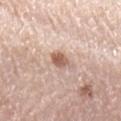Notes:
• biopsy status · no biopsy performed (imaged during a skin exam)
• acquisition · ~15 mm tile from a whole-body skin photo
• patient · female, in their mid-40s
• diameter · ≈2.5 mm
• location · the left forearm